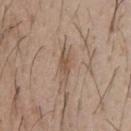Clinical impression:
No biopsy was performed on this lesion — it was imaged during a full skin examination and was not determined to be concerning.
Image and clinical context:
A male subject approximately 30 years of age. Located on the upper back. The total-body-photography lesion software estimated an area of roughly 5.5 mm², a shape eccentricity near 0.9, and a shape-asymmetry score of about 0.25 (0 = symmetric). It also reported a color-variation rating of about 2.5/10 and peripheral color asymmetry of about 0.5. The recorded lesion diameter is about 4 mm. The tile uses white-light illumination. A 15 mm close-up extracted from a 3D total-body photography capture.